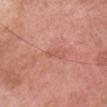Notes:
- biopsy status · catalogued during a skin exam; not biopsied
- anatomic site · the head or neck
- patient · female, aged approximately 50
- lighting · white-light illumination
- image · 15 mm crop, total-body photography
- image-analysis metrics · an outline eccentricity of about 0.8 (0 = round, 1 = elongated) and a symmetry-axis asymmetry near 0.35